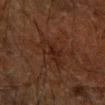Clinical impression: The lesion was tiled from a total-body skin photograph and was not biopsied. Image and clinical context: Automated tile analysis of the lesion measured a lesion area of about 7.5 mm², an eccentricity of roughly 0.85, and two-axis asymmetry of about 0.3. The software also gave an average lesion color of about L≈17 a*≈15 b*≈20 (CIELAB), about 4 CIELAB-L* units darker than the surrounding skin, and a normalized lesion–skin contrast near 6. The software also gave a border-irregularity rating of about 4/10, a within-lesion color-variation index near 3/10, and a peripheral color-asymmetry measure near 1. A 15 mm close-up extracted from a 3D total-body photography capture. This is a cross-polarized tile. A male patient aged 58 to 62. The lesion is located on the right forearm.